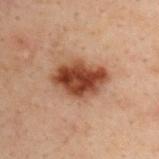Findings:
- workup: imaged on a skin check; not biopsied
- tile lighting: cross-polarized illumination
- automated metrics: a lesion area of about 17 mm², an eccentricity of roughly 0.7, and a symmetry-axis asymmetry near 0.15
- subject: male, aged around 30
- image: ~15 mm crop, total-body skin-cancer survey
- lesion diameter: about 5.5 mm
- anatomic site: the back